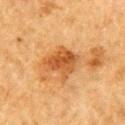{
  "biopsy_status": "not biopsied; imaged during a skin examination",
  "lesion_size": {
    "long_diameter_mm_approx": 4.0
  },
  "image": {
    "source": "total-body photography crop",
    "field_of_view_mm": 15
  },
  "patient": {
    "sex": "male",
    "age_approx": "85+"
  },
  "automated_metrics": {
    "border_irregularity_0_10": 3.5,
    "color_variation_0_10": 4.5,
    "peripheral_color_asymmetry": 1.5,
    "nevus_likeness_0_100": 90,
    "lesion_detection_confidence_0_100": 100
  },
  "site": "right upper arm",
  "lighting": "cross-polarized"
}